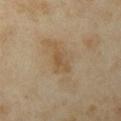follow-up: catalogued during a skin exam; not biopsied
TBP lesion metrics: about 6 CIELAB-L* units darker than the surrounding skin and a lesion-to-skin contrast of about 5.5 (normalized; higher = more distinct); internal color variation of about 2 on a 0–10 scale; a nevus-likeness score of about 0/100 and lesion-presence confidence of about 100/100
anatomic site: the left upper arm
tile lighting: cross-polarized illumination
diameter: about 4 mm
image: ~15 mm crop, total-body skin-cancer survey
subject: female, in their mid- to late 30s